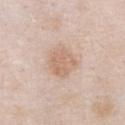Part of a total-body skin-imaging series; this lesion was reviewed on a skin check and was not flagged for biopsy. The tile uses white-light illumination. A close-up tile cropped from a whole-body skin photograph, about 15 mm across. The subject is a male approximately 55 years of age. The lesion is on the chest.